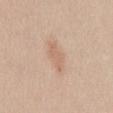No biopsy was performed on this lesion — it was imaged during a full skin examination and was not determined to be concerning. A close-up tile cropped from a whole-body skin photograph, about 15 mm across. Automated tile analysis of the lesion measured an area of roughly 6 mm² and two-axis asymmetry of about 0.25. It also reported a color-variation rating of about 1.5/10. The software also gave a classifier nevus-likeness of about 0/100 and lesion-presence confidence of about 100/100. Approximately 4 mm at its widest. A male subject approximately 45 years of age. The lesion is on the abdomen. The tile uses white-light illumination.